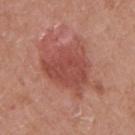From the right upper arm. A female patient, approximately 40 years of age. Cropped from a whole-body photographic skin survey; the tile spans about 15 mm.